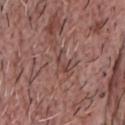Impression:
Imaged during a routine full-body skin examination; the lesion was not biopsied and no histopathology is available.
Context:
A region of skin cropped from a whole-body photographic capture, roughly 15 mm wide. An algorithmic analysis of the crop reported a footprint of about 2.5 mm², an outline eccentricity of about 0.9 (0 = round, 1 = elongated), and a shape-asymmetry score of about 0.6 (0 = symmetric). The software also gave an average lesion color of about L≈43 a*≈21 b*≈22 (CIELAB) and a lesion–skin lightness drop of about 8. The software also gave a classifier nevus-likeness of about 0/100 and a detector confidence of about 70 out of 100 that the crop contains a lesion. A male subject, about 65 years old. This is a white-light tile. On the chest.